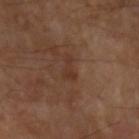Case summary:
– workup: no biopsy performed (imaged during a skin exam)
– lesion diameter: about 3 mm
– image source: ~15 mm tile from a whole-body skin photo
– automated lesion analysis: a lesion area of about 3 mm², an outline eccentricity of about 0.95 (0 = round, 1 = elongated), and two-axis asymmetry of about 0.55
– body site: the left upper arm
– lighting: cross-polarized illumination